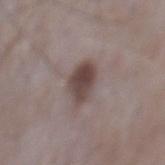Clinical impression: This lesion was catalogued during total-body skin photography and was not selected for biopsy. Background: A 15 mm crop from a total-body photograph taken for skin-cancer surveillance. Automated image analysis of the tile measured a footprint of about 8 mm², a shape eccentricity near 0.75, and two-axis asymmetry of about 0.2. The analysis additionally found an average lesion color of about L≈43 a*≈14 b*≈17 (CIELAB), roughly 13 lightness units darker than nearby skin, and a lesion-to-skin contrast of about 9.5 (normalized; higher = more distinct). The software also gave a border-irregularity rating of about 2/10, internal color variation of about 4.5 on a 0–10 scale, and radial color variation of about 1.5. And it measured a nevus-likeness score of about 85/100 and lesion-presence confidence of about 100/100. The subject is a male in their mid- to late 60s. Imaged with white-light lighting. From the mid back. About 4 mm across.